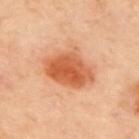follow-up = imaged on a skin check; not biopsied | tile lighting = cross-polarized | acquisition = 15 mm crop, total-body photography | site = the upper back | size = ~6 mm (longest diameter).